A lesion tile, about 15 mm wide, cut from a 3D total-body photograph; a male subject, in their mid-40s; from the left lower leg — 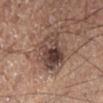* lesion size: about 5 mm
* lighting: cross-polarized illumination
* diagnosis: an atypical melanocytic neoplasm — a lesion of indeterminate malignant potential